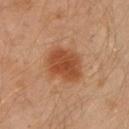Findings:
– image: ~15 mm tile from a whole-body skin photo
– tile lighting: cross-polarized illumination
– patient: male, aged approximately 30
– anatomic site: the left arm
– size: ~5 mm (longest diameter)